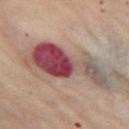Part of a total-body skin-imaging series; this lesion was reviewed on a skin check and was not flagged for biopsy. The lesion is located on the leg. The tile uses cross-polarized illumination. The subject is a female approximately 60 years of age. A roughly 15 mm field-of-view crop from a total-body skin photograph. The total-body-photography lesion software estimated a border-irregularity rating of about 5.5/10, internal color variation of about 10 on a 0–10 scale, and radial color variation of about 6. The software also gave a nevus-likeness score of about 0/100 and a lesion-detection confidence of about 100/100. The lesion's longest dimension is about 10 mm.